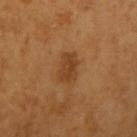Captured during whole-body skin photography for melanoma surveillance; the lesion was not biopsied.
Captured under cross-polarized illumination.
Cropped from a whole-body photographic skin survey; the tile spans about 15 mm.
About 3.5 mm across.
Located on the right upper arm.
The patient is a male in their mid- to late 60s.